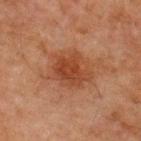workup: catalogued during a skin exam; not biopsied
imaging modality: 15 mm crop, total-body photography
image-analysis metrics: a mean CIELAB color near L≈35 a*≈22 b*≈29 and a lesion-to-skin contrast of about 7.5 (normalized; higher = more distinct); a border-irregularity index near 3/10; a classifier nevus-likeness of about 45/100
body site: the front of the torso
subject: male, roughly 65 years of age
lesion diameter: ~5 mm (longest diameter)